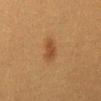Clinical impression: The lesion was tiled from a total-body skin photograph and was not biopsied. Background: A lesion tile, about 15 mm wide, cut from a 3D total-body photograph. Captured under cross-polarized illumination. Automated tile analysis of the lesion measured a lesion color around L≈39 a*≈18 b*≈32 in CIELAB, roughly 7 lightness units darker than nearby skin, and a normalized lesion–skin contrast near 6.5. The lesion is located on the abdomen. Approximately 3.5 mm at its widest. A female subject aged around 40.